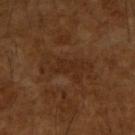Assessment: The lesion was photographed on a routine skin check and not biopsied; there is no pathology result. Acquisition and patient details: Located on the arm. The lesion's longest dimension is about 6.5 mm. Imaged with cross-polarized lighting. A male subject, in their mid-60s. Cropped from a total-body skin-imaging series; the visible field is about 15 mm.